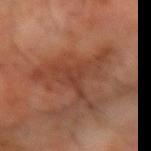Part of a total-body skin-imaging series; this lesion was reviewed on a skin check and was not flagged for biopsy. A male subject roughly 60 years of age. This image is a 15 mm lesion crop taken from a total-body photograph. The lesion is on the right forearm.